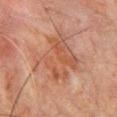Impression: The lesion was photographed on a routine skin check and not biopsied; there is no pathology result. Clinical summary: A roughly 15 mm field-of-view crop from a total-body skin photograph. The subject is a male roughly 65 years of age. About 7 mm across. Captured under cross-polarized illumination. The lesion is located on the chest. Automated image analysis of the tile measured a footprint of about 28 mm², an outline eccentricity of about 0.25 (0 = round, 1 = elongated), and a symmetry-axis asymmetry near 0.35. And it measured a lesion color around L≈44 a*≈19 b*≈27 in CIELAB and a normalized lesion–skin contrast near 5.5. And it measured an automated nevus-likeness rating near 0 out of 100 and a lesion-detection confidence of about 100/100.